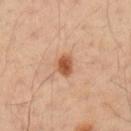biopsy_status: not biopsied; imaged during a skin examination
lighting: cross-polarized
automated_metrics:
  area_mm2_approx: 4.0
  shape_asymmetry: 0.25
  border_irregularity_0_10: 2.0
  nevus_likeness_0_100: 100
  lesion_detection_confidence_0_100: 100
patient:
  sex: male
  age_approx: 50
site: left upper arm
image:
  source: total-body photography crop
  field_of_view_mm: 15